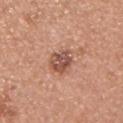  biopsy_status: not biopsied; imaged during a skin examination
  image:
    source: total-body photography crop
    field_of_view_mm: 15
  patient:
    sex: male
    age_approx: 30
  site: chest
  lighting: white-light
  lesion_size:
    long_diameter_mm_approx: 3.0